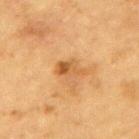Recorded during total-body skin imaging; not selected for excision or biopsy. About 3.5 mm across. Automated image analysis of the tile measured an outline eccentricity of about 0.85 (0 = round, 1 = elongated) and a symmetry-axis asymmetry near 0.3. The analysis additionally found a lesion color around L≈50 a*≈21 b*≈40 in CIELAB, a lesion–skin lightness drop of about 9, and a normalized lesion–skin contrast near 7. The software also gave a peripheral color-asymmetry measure near 2. The analysis additionally found a nevus-likeness score of about 5/100 and a detector confidence of about 100 out of 100 that the crop contains a lesion. A 15 mm close-up extracted from a 3D total-body photography capture. The lesion is located on the upper back. The subject is a male about 85 years old or older.